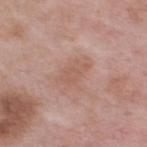notes = no biopsy performed (imaged during a skin exam)
size = about 3.5 mm
TBP lesion metrics = a footprint of about 5.5 mm², a shape eccentricity near 0.85, and two-axis asymmetry of about 0.3; border irregularity of about 3 on a 0–10 scale and a peripheral color-asymmetry measure near 0.5
anatomic site = the upper back
subject = male, roughly 55 years of age
illumination = white-light
acquisition = total-body-photography crop, ~15 mm field of view